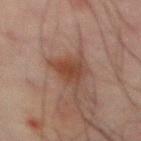The lesion was photographed on a routine skin check and not biopsied; there is no pathology result.
The total-body-photography lesion software estimated internal color variation of about 2.5 on a 0–10 scale.
The lesion is on the mid back.
A male subject aged 63–67.
A 15 mm close-up extracted from a 3D total-body photography capture.
Approximately 3 mm at its widest.
The tile uses cross-polarized illumination.